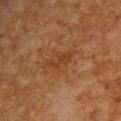Impression:
The lesion was tiled from a total-body skin photograph and was not biopsied.
Context:
The lesion's longest dimension is about 3.5 mm. On the upper back. The tile uses cross-polarized illumination. A 15 mm close-up tile from a total-body photography series done for melanoma screening. A male patient in their mid-60s.